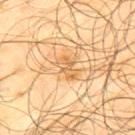No biopsy was performed on this lesion — it was imaged during a full skin examination and was not determined to be concerning.
The lesion is located on the upper back.
A close-up tile cropped from a whole-body skin photograph, about 15 mm across.
Imaged with cross-polarized lighting.
Longest diameter approximately 3 mm.
A male subject aged around 65.